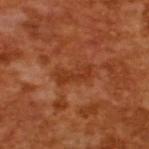{
  "biopsy_status": "not biopsied; imaged during a skin examination",
  "image": {
    "source": "total-body photography crop",
    "field_of_view_mm": 15
  },
  "lighting": "cross-polarized",
  "lesion_size": {
    "long_diameter_mm_approx": 4.0
  },
  "patient": {
    "sex": "male",
    "age_approx": 65
  }
}